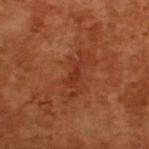Q: Was this lesion biopsied?
A: imaged on a skin check; not biopsied
Q: How was this image acquired?
A: total-body-photography crop, ~15 mm field of view
Q: Illumination type?
A: cross-polarized
Q: Lesion size?
A: about 3 mm
Q: What are the patient's age and sex?
A: male, aged around 65